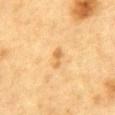{
  "biopsy_status": "not biopsied; imaged during a skin examination",
  "lighting": "cross-polarized",
  "lesion_size": {
    "long_diameter_mm_approx": 2.5
  },
  "image": {
    "source": "total-body photography crop",
    "field_of_view_mm": 15
  },
  "site": "abdomen",
  "patient": {
    "sex": "male",
    "age_approx": "85+"
  },
  "automated_metrics": {
    "color_variation_0_10": 1.0,
    "nevus_likeness_0_100": 0,
    "lesion_detection_confidence_0_100": 100
  }
}